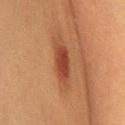lesion diameter: about 6 mm
image-analysis metrics: an area of roughly 10 mm² and an eccentricity of roughly 0.95; a mean CIELAB color near L≈37 a*≈22 b*≈29 and roughly 9 lightness units darker than nearby skin; an automated nevus-likeness rating near 100 out of 100
patient: female, aged approximately 55
lighting: cross-polarized illumination
location: the chest
acquisition: 15 mm crop, total-body photography